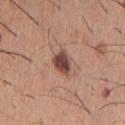workup = catalogued during a skin exam; not biopsied | tile lighting = white-light illumination | patient = male, aged 58 to 62 | automated lesion analysis = a footprint of about 5.5 mm² and a symmetry-axis asymmetry near 0.25; a border-irregularity index near 2.5/10, internal color variation of about 3.5 on a 0–10 scale, and peripheral color asymmetry of about 1 | imaging modality = ~15 mm crop, total-body skin-cancer survey | lesion diameter = ~3 mm (longest diameter) | site = the chest.